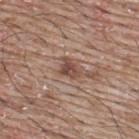biopsy_status: not biopsied; imaged during a skin examination
patient:
  sex: male
  age_approx: 65
image:
  source: total-body photography crop
  field_of_view_mm: 15
lighting: white-light
site: upper back
lesion_size:
  long_diameter_mm_approx: 3.0